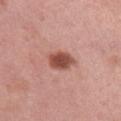Assessment:
Part of a total-body skin-imaging series; this lesion was reviewed on a skin check and was not flagged for biopsy.
Background:
A close-up tile cropped from a whole-body skin photograph, about 15 mm across. The lesion is located on the left thigh. A female subject, aged around 50. The lesion-visualizer software estimated a border-irregularity index near 1.5/10, internal color variation of about 3.5 on a 0–10 scale, and peripheral color asymmetry of about 1. This is a white-light tile.